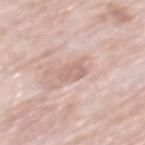The lesion was tiled from a total-body skin photograph and was not biopsied. A 15 mm crop from a total-body photograph taken for skin-cancer surveillance. The lesion is located on the mid back. A male subject aged 78 to 82. An algorithmic analysis of the crop reported a mean CIELAB color near L≈65 a*≈18 b*≈24, roughly 8 lightness units darker than nearby skin, and a normalized border contrast of about 5. This is a white-light tile. Longest diameter approximately 3 mm.